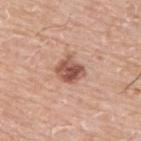This lesion was catalogued during total-body skin photography and was not selected for biopsy.
This is a white-light tile.
The subject is a male roughly 75 years of age.
The lesion is on the back.
Longest diameter approximately 3 mm.
A region of skin cropped from a whole-body photographic capture, roughly 15 mm wide.
Automated tile analysis of the lesion measured an eccentricity of roughly 0.45 and a shape-asymmetry score of about 0.25 (0 = symmetric). The analysis additionally found a mean CIELAB color near L≈54 a*≈23 b*≈28. The analysis additionally found a peripheral color-asymmetry measure near 2. And it measured a nevus-likeness score of about 80/100.